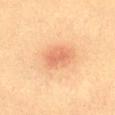  biopsy_status: not biopsied; imaged during a skin examination
  lighting: cross-polarized
  automated_metrics:
    area_mm2_approx: 5.0
    eccentricity: 0.6
    shape_asymmetry: 0.3
    border_irregularity_0_10: 2.5
    peripheral_color_asymmetry: 0.5
    nevus_likeness_0_100: 20
    lesion_detection_confidence_0_100: 100
  patient:
    sex: female
    age_approx: 55
  image:
    source: total-body photography crop
    field_of_view_mm: 15
  lesion_size:
    long_diameter_mm_approx: 3.0
  site: abdomen